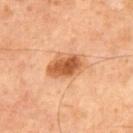<record>
  <patient>
    <sex>male</sex>
    <age_approx>70</age_approx>
  </patient>
  <image>
    <source>total-body photography crop</source>
    <field_of_view_mm>15</field_of_view_mm>
  </image>
  <site>chest</site>
</record>